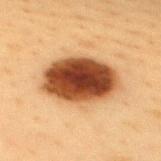Located on the upper back. A female subject aged approximately 40. Measured at roughly 7.5 mm in maximum diameter. This image is a 15 mm lesion crop taken from a total-body photograph.